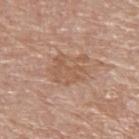{
  "biopsy_status": "not biopsied; imaged during a skin examination",
  "site": "left upper arm",
  "patient": {
    "sex": "male",
    "age_approx": 70
  },
  "image": {
    "source": "total-body photography crop",
    "field_of_view_mm": 15
  },
  "lighting": "white-light"
}